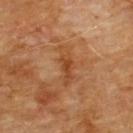– biopsy status: catalogued during a skin exam; not biopsied
– image source: ~15 mm tile from a whole-body skin photo
– lesion size: about 5 mm
– location: the chest
– image-analysis metrics: a footprint of about 7 mm², a shape eccentricity near 0.95, and two-axis asymmetry of about 0.3; a mean CIELAB color near L≈45 a*≈23 b*≈37 and a normalized lesion–skin contrast near 6.5; border irregularity of about 4.5 on a 0–10 scale, a color-variation rating of about 3.5/10, and peripheral color asymmetry of about 1; a nevus-likeness score of about 0/100
– lighting: cross-polarized
– patient: male, aged 58–62